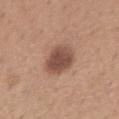The lesion was tiled from a total-body skin photograph and was not biopsied.